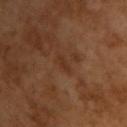Q: Was this lesion biopsied?
A: no biopsy performed (imaged during a skin exam)
Q: Illumination type?
A: cross-polarized illumination
Q: Lesion size?
A: about 2.5 mm
Q: Patient demographics?
A: male, aged approximately 65
Q: How was this image acquired?
A: total-body-photography crop, ~15 mm field of view